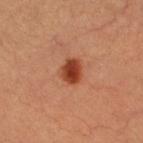| key | value |
|---|---|
| image source | ~15 mm tile from a whole-body skin photo |
| patient | female, aged approximately 45 |
| tile lighting | cross-polarized |
| lesion diameter | ≈3 mm |
| site | the left leg |